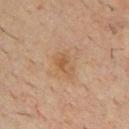Impression: Imaged during a routine full-body skin examination; the lesion was not biopsied and no histopathology is available. Clinical summary: A male patient, aged around 35. On the chest. A 15 mm close-up extracted from a 3D total-body photography capture.